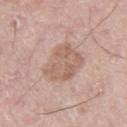workup = no biopsy performed (imaged during a skin exam)
automated metrics = a footprint of about 16 mm²; a mean CIELAB color near L≈61 a*≈18 b*≈26, about 8 CIELAB-L* units darker than the surrounding skin, and a normalized border contrast of about 5.5; border irregularity of about 2.5 on a 0–10 scale, internal color variation of about 3.5 on a 0–10 scale, and radial color variation of about 1; a detector confidence of about 100 out of 100 that the crop contains a lesion
site = the right thigh
diameter = ~4.5 mm (longest diameter)
patient = male, aged 68–72
imaging modality = 15 mm crop, total-body photography
illumination = white-light illumination